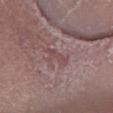Background: An algorithmic analysis of the crop reported a footprint of about 4 mm², an eccentricity of roughly 0.85, and a symmetry-axis asymmetry near 0.4. It also reported a mean CIELAB color near L≈47 a*≈20 b*≈18, roughly 6 lightness units darker than nearby skin, and a normalized lesion–skin contrast near 5. Captured under white-light illumination. From the left lower leg. The lesion's longest dimension is about 3 mm. The subject is a male in their mid- to late 40s. A 15 mm close-up extracted from a 3D total-body photography capture.